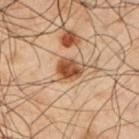Assessment: This lesion was catalogued during total-body skin photography and was not selected for biopsy. Clinical summary: This is a cross-polarized tile. A lesion tile, about 15 mm wide, cut from a 3D total-body photograph. A male subject, roughly 50 years of age. The lesion is located on the left upper arm. Longest diameter approximately 3 mm.